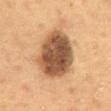Findings:
* workup: total-body-photography surveillance lesion; no biopsy
* size: ~7 mm (longest diameter)
* subject: male, in their 60s
* image-analysis metrics: border irregularity of about 1.5 on a 0–10 scale, a color-variation rating of about 6/10, and a peripheral color-asymmetry measure near 1.5
* anatomic site: the abdomen
* acquisition: 15 mm crop, total-body photography